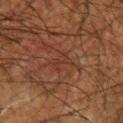notes: catalogued during a skin exam; not biopsied | subject: male, approximately 65 years of age | illumination: cross-polarized illumination | automated lesion analysis: a lesion color around L≈28 a*≈18 b*≈23 in CIELAB and roughly 4 lightness units darker than nearby skin | location: the left forearm | imaging modality: ~15 mm crop, total-body skin-cancer survey.